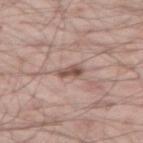workup: no biopsy performed (imaged during a skin exam); lesion diameter: about 2.5 mm; location: the leg; tile lighting: white-light illumination; imaging modality: ~15 mm crop, total-body skin-cancer survey; patient: male, in their mid-50s.